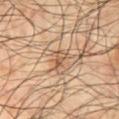notes = no biopsy performed (imaged during a skin exam)
size = ~2.5 mm (longest diameter)
lighting = cross-polarized illumination
image = 15 mm crop, total-body photography
patient = male, in their mid- to late 60s
body site = the abdomen
automated lesion analysis = a border-irregularity index near 3/10; a nevus-likeness score of about 0/100 and a lesion-detection confidence of about 80/100